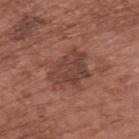Impression: Recorded during total-body skin imaging; not selected for excision or biopsy. Image and clinical context: Cropped from a whole-body photographic skin survey; the tile spans about 15 mm. The tile uses white-light illumination. From the upper back. The subject is a male aged 73–77. An algorithmic analysis of the crop reported a nevus-likeness score of about 20/100 and lesion-presence confidence of about 100/100. The lesion's longest dimension is about 5 mm.